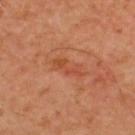| field | value |
|---|---|
| workup | no biopsy performed (imaged during a skin exam) |
| site | the upper back |
| acquisition | 15 mm crop, total-body photography |
| size | ≈4 mm |
| image-analysis metrics | a lesion area of about 5 mm², a shape eccentricity near 0.95, and a shape-asymmetry score of about 0.45 (0 = symmetric); a mean CIELAB color near L≈49 a*≈29 b*≈36 and a lesion–skin lightness drop of about 7; a color-variation rating of about 2/10 |
| illumination | cross-polarized |
| subject | male, about 50 years old |